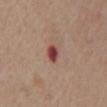<lesion>
  <biopsy_status>not biopsied; imaged during a skin examination</biopsy_status>
  <image>
    <source>total-body photography crop</source>
    <field_of_view_mm>15</field_of_view_mm>
  </image>
  <site>front of the torso</site>
  <lesion_size>
    <long_diameter_mm_approx>2.5</long_diameter_mm_approx>
  </lesion_size>
  <patient>
    <sex>male</sex>
    <age_approx>75</age_approx>
  </patient>
</lesion>